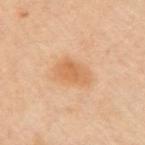Q: Was this lesion biopsied?
A: total-body-photography surveillance lesion; no biopsy
Q: How was the tile lit?
A: cross-polarized illumination
Q: Lesion location?
A: the left arm
Q: Lesion size?
A: ~4 mm (longest diameter)
Q: What is the imaging modality?
A: ~15 mm crop, total-body skin-cancer survey
Q: Who is the patient?
A: female, aged 38 to 42
Q: What did automated image analysis measure?
A: a nevus-likeness score of about 75/100 and lesion-presence confidence of about 100/100